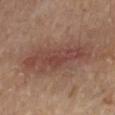Assessment:
This lesion was catalogued during total-body skin photography and was not selected for biopsy.
Image and clinical context:
Captured under cross-polarized illumination. On the arm. A female patient, aged approximately 65. A roughly 15 mm field-of-view crop from a total-body skin photograph.